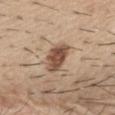Clinical impression: This lesion was catalogued during total-body skin photography and was not selected for biopsy. Acquisition and patient details: The lesion is located on the front of the torso. The tile uses white-light illumination. A lesion tile, about 15 mm wide, cut from a 3D total-body photograph. The lesion-visualizer software estimated an area of roughly 8.5 mm² and an outline eccentricity of about 0.7 (0 = round, 1 = elongated). A male subject aged around 60.